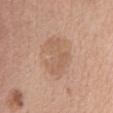Captured during whole-body skin photography for melanoma surveillance; the lesion was not biopsied.
The tile uses white-light illumination.
On the chest.
A female patient, aged around 55.
Cropped from a total-body skin-imaging series; the visible field is about 15 mm.